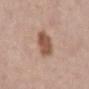| feature | finding |
|---|---|
| biopsy status | total-body-photography surveillance lesion; no biopsy |
| subject | male, in their mid- to late 50s |
| lesion size | ~3.5 mm (longest diameter) |
| image | 15 mm crop, total-body photography |
| body site | the abdomen |
| tile lighting | white-light |
| image-analysis metrics | a lesion area of about 9 mm², an outline eccentricity of about 0.55 (0 = round, 1 = elongated), and a shape-asymmetry score of about 0.15 (0 = symmetric); a mean CIELAB color near L≈53 a*≈20 b*≈28 and a normalized lesion–skin contrast near 9; a border-irregularity rating of about 1.5/10, internal color variation of about 3.5 on a 0–10 scale, and radial color variation of about 1 |